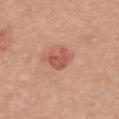* biopsy status: imaged on a skin check; not biopsied
* anatomic site: the front of the torso
* automated metrics: a lesion–skin lightness drop of about 10; a nevus-likeness score of about 65/100
* subject: female, aged around 60
* image source: total-body-photography crop, ~15 mm field of view
* size: ≈3.5 mm
* illumination: white-light illumination